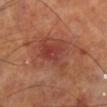Context:
Automated tile analysis of the lesion measured a lesion–skin lightness drop of about 8. The software also gave a border-irregularity rating of about 5.5/10 and internal color variation of about 5.5 on a 0–10 scale. The software also gave a nevus-likeness score of about 50/100 and a lesion-detection confidence of about 100/100. On the left lower leg. The tile uses cross-polarized illumination. The subject is a male aged 68 to 72. Measured at roughly 9 mm in maximum diameter. This image is a 15 mm lesion crop taken from a total-body photograph.